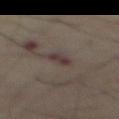biopsy status: imaged on a skin check; not biopsied
illumination: cross-polarized
acquisition: ~15 mm tile from a whole-body skin photo
body site: the abdomen
lesion size: ≈2.5 mm
patient: male, roughly 55 years of age